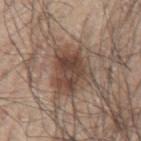<record>
<biopsy_status>not biopsied; imaged during a skin examination</biopsy_status>
</record>